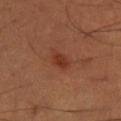notes=no biopsy performed (imaged during a skin exam); lesion size=~3 mm (longest diameter); location=the right lower leg; image=total-body-photography crop, ~15 mm field of view; subject=male, approximately 50 years of age; tile lighting=cross-polarized illumination.